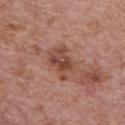| feature | finding |
|---|---|
| notes | total-body-photography surveillance lesion; no biopsy |
| location | the chest |
| automated metrics | a lesion color around L≈47 a*≈22 b*≈27 in CIELAB and roughly 10 lightness units darker than nearby skin; a border-irregularity rating of about 3.5/10, internal color variation of about 5.5 on a 0–10 scale, and radial color variation of about 2; a classifier nevus-likeness of about 5/100 and a detector confidence of about 100 out of 100 that the crop contains a lesion |
| image source | ~15 mm crop, total-body skin-cancer survey |
| illumination | white-light |
| subject | male, aged around 70 |
| size | ~4.5 mm (longest diameter) |